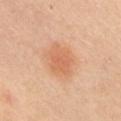Assessment: Imaged during a routine full-body skin examination; the lesion was not biopsied and no histopathology is available. Image and clinical context: The recorded lesion diameter is about 4.5 mm. The lesion is on the right upper arm. A female patient approximately 40 years of age. A region of skin cropped from a whole-body photographic capture, roughly 15 mm wide. This is a cross-polarized tile.